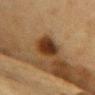No biopsy was performed on this lesion — it was imaged during a full skin examination and was not determined to be concerning.
The tile uses cross-polarized illumination.
The lesion's longest dimension is about 4 mm.
The subject is a female aged approximately 55.
On the front of the torso.
This image is a 15 mm lesion crop taken from a total-body photograph.